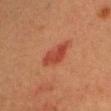No biopsy was performed on this lesion — it was imaged during a full skin examination and was not determined to be concerning. A 15 mm close-up extracted from a 3D total-body photography capture. Located on the head or neck. A male subject about 40 years old. This is a cross-polarized tile.